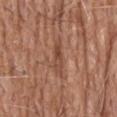Q: Was this lesion biopsied?
A: imaged on a skin check; not biopsied